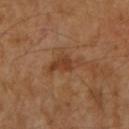Recorded during total-body skin imaging; not selected for excision or biopsy. Automated tile analysis of the lesion measured an automated nevus-likeness rating near 0 out of 100 and lesion-presence confidence of about 100/100. The lesion's longest dimension is about 4.5 mm. Cropped from a whole-body photographic skin survey; the tile spans about 15 mm. Imaged with cross-polarized lighting. A female subject, aged around 70. The lesion is located on the left forearm.